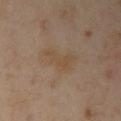Impression: The lesion was tiled from a total-body skin photograph and was not biopsied. Acquisition and patient details: A female patient approximately 40 years of age. The lesion's longest dimension is about 4.5 mm. Captured under cross-polarized illumination. The lesion is on the left arm. The total-body-photography lesion software estimated an area of roughly 8.5 mm², a shape eccentricity near 0.85, and a shape-asymmetry score of about 0.4 (0 = symmetric). And it measured a mean CIELAB color near L≈49 a*≈14 b*≈29, a lesion–skin lightness drop of about 5, and a normalized border contrast of about 5. A 15 mm crop from a total-body photograph taken for skin-cancer surveillance.